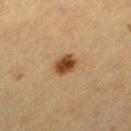<record>
<biopsy_status>not biopsied; imaged during a skin examination</biopsy_status>
<lighting>cross-polarized</lighting>
<automated_metrics>
  <cielab_L>36</cielab_L>
  <cielab_a>18</cielab_a>
  <cielab_b>31</cielab_b>
  <vs_skin_contrast_norm>11.5</vs_skin_contrast_norm>
  <lesion_detection_confidence_0_100>100</lesion_detection_confidence_0_100>
</automated_metrics>
<site>left thigh</site>
<patient>
  <sex>female</sex>
  <age_approx>55</age_approx>
</patient>
<image>
  <source>total-body photography crop</source>
  <field_of_view_mm>15</field_of_view_mm>
</image>
</record>